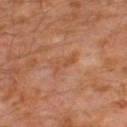follow-up = imaged on a skin check; not biopsied
body site = the left thigh
image = ~15 mm tile from a whole-body skin photo
illumination = cross-polarized
patient = male, approximately 30 years of age
diameter = ≈3 mm